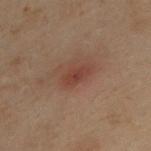Q: Was this lesion biopsied?
A: total-body-photography surveillance lesion; no biopsy
Q: How large is the lesion?
A: about 3 mm
Q: What kind of image is this?
A: total-body-photography crop, ~15 mm field of view
Q: Lesion location?
A: the upper back
Q: Illumination type?
A: cross-polarized illumination
Q: What are the patient's age and sex?
A: male, roughly 30 years of age